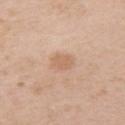follow-up = total-body-photography surveillance lesion; no biopsy | lesion size = ≈2.5 mm | tile lighting = white-light | image source = ~15 mm crop, total-body skin-cancer survey | body site = the left upper arm | patient = female, roughly 40 years of age.